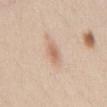<case>
  <image>
    <source>total-body photography crop</source>
    <field_of_view_mm>15</field_of_view_mm>
  </image>
  <patient>
    <sex>male</sex>
    <age_approx>25</age_approx>
  </patient>
  <site>abdomen</site>
</case>